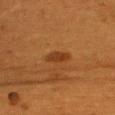Case summary:
• biopsy status — catalogued during a skin exam; not biopsied
• patient — female, in their mid-50s
• lesion diameter — about 3.5 mm
• image source — ~15 mm tile from a whole-body skin photo
• body site — the front of the torso
• illumination — cross-polarized illumination